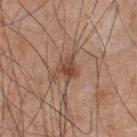{"biopsy_status": "not biopsied; imaged during a skin examination", "patient": {"sex": "male", "age_approx": 55}, "site": "chest", "image": {"source": "total-body photography crop", "field_of_view_mm": 15}}